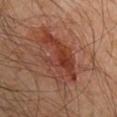The lesion was tiled from a total-body skin photograph and was not biopsied. The lesion's longest dimension is about 8 mm. A male patient aged 53–57. An algorithmic analysis of the crop reported an area of roughly 21 mm², an eccentricity of roughly 0.9, and two-axis asymmetry of about 0.35. And it measured an average lesion color of about L≈39 a*≈25 b*≈28 (CIELAB), a lesion–skin lightness drop of about 9, and a lesion-to-skin contrast of about 7.5 (normalized; higher = more distinct). And it measured a nevus-likeness score of about 15/100 and lesion-presence confidence of about 100/100. A lesion tile, about 15 mm wide, cut from a 3D total-body photograph. On the right upper arm. Captured under cross-polarized illumination.